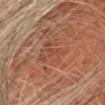Clinical impression:
Imaged during a routine full-body skin examination; the lesion was not biopsied and no histopathology is available.
Context:
The total-body-photography lesion software estimated a footprint of about 9.5 mm², an outline eccentricity of about 0.85 (0 = round, 1 = elongated), and a symmetry-axis asymmetry near 0.45. The software also gave a mean CIELAB color near L≈41 a*≈22 b*≈27, roughly 6 lightness units darker than nearby skin, and a normalized lesion–skin contrast near 5. And it measured a classifier nevus-likeness of about 0/100 and a detector confidence of about 65 out of 100 that the crop contains a lesion. A male patient, aged 63 to 67. The lesion is located on the head or neck. A roughly 15 mm field-of-view crop from a total-body skin photograph.